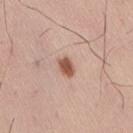| feature | finding |
|---|---|
| follow-up | catalogued during a skin exam; not biopsied |
| acquisition | 15 mm crop, total-body photography |
| site | the right thigh |
| automated metrics | a nevus-likeness score of about 95/100 |
| illumination | white-light |
| subject | male, aged around 40 |